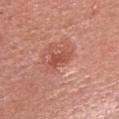Part of a total-body skin-imaging series; this lesion was reviewed on a skin check and was not flagged for biopsy.
A female patient approximately 35 years of age.
A lesion tile, about 15 mm wide, cut from a 3D total-body photograph.
The lesion is on the head or neck.